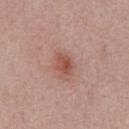Clinical impression: Captured during whole-body skin photography for melanoma surveillance; the lesion was not biopsied. Image and clinical context: Located on the front of the torso. A male patient aged 58–62. The tile uses white-light illumination. The recorded lesion diameter is about 3 mm. An algorithmic analysis of the crop reported an area of roughly 4.5 mm², a shape eccentricity near 0.7, and a shape-asymmetry score of about 0.2 (0 = symmetric). The software also gave a mean CIELAB color near L≈52 a*≈25 b*≈26, about 10 CIELAB-L* units darker than the surrounding skin, and a lesion-to-skin contrast of about 7.5 (normalized; higher = more distinct). The analysis additionally found an automated nevus-likeness rating near 70 out of 100 and a lesion-detection confidence of about 100/100. This image is a 15 mm lesion crop taken from a total-body photograph.